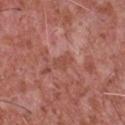Q: Was a biopsy performed?
A: total-body-photography surveillance lesion; no biopsy
Q: Lesion size?
A: ≈2.5 mm
Q: Where on the body is the lesion?
A: the chest
Q: What lighting was used for the tile?
A: white-light
Q: What is the imaging modality?
A: ~15 mm tile from a whole-body skin photo
Q: What are the patient's age and sex?
A: male, roughly 45 years of age
Q: Automated lesion metrics?
A: an area of roughly 3 mm² and two-axis asymmetry of about 0.3; a border-irregularity rating of about 3/10, internal color variation of about 1.5 on a 0–10 scale, and radial color variation of about 0.5